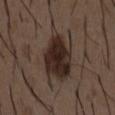Q: Was this lesion biopsied?
A: imaged on a skin check; not biopsied
Q: How was the tile lit?
A: white-light
Q: Patient demographics?
A: male, approximately 50 years of age
Q: What kind of image is this?
A: 15 mm crop, total-body photography
Q: Where on the body is the lesion?
A: the chest
Q: Lesion size?
A: about 6.5 mm